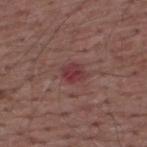No biopsy was performed on this lesion — it was imaged during a full skin examination and was not determined to be concerning.
A male patient aged around 55.
An algorithmic analysis of the crop reported an area of roughly 4.5 mm², an outline eccentricity of about 0.55 (0 = round, 1 = elongated), and two-axis asymmetry of about 0.2. And it measured a lesion–skin lightness drop of about 9 and a lesion-to-skin contrast of about 7.5 (normalized; higher = more distinct). The analysis additionally found an automated nevus-likeness rating near 5 out of 100 and a detector confidence of about 100 out of 100 that the crop contains a lesion.
This is a white-light tile.
A 15 mm crop from a total-body photograph taken for skin-cancer surveillance.
From the upper back.
The recorded lesion diameter is about 2.5 mm.